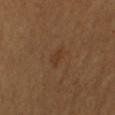A male subject, aged 63–67.
Automated tile analysis of the lesion measured a shape eccentricity near 0.75 and two-axis asymmetry of about 0.35. And it measured a border-irregularity rating of about 3.5/10, internal color variation of about 1 on a 0–10 scale, and radial color variation of about 0.5.
A close-up tile cropped from a whole-body skin photograph, about 15 mm across.
The lesion is located on the right upper arm.
Measured at roughly 2.5 mm in maximum diameter.